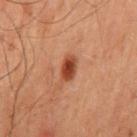Clinical impression: Recorded during total-body skin imaging; not selected for excision or biopsy. Clinical summary: From the mid back. Captured under cross-polarized illumination. This image is a 15 mm lesion crop taken from a total-body photograph. A male patient roughly 60 years of age. About 2.5 mm across.